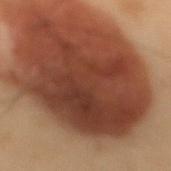| key | value |
|---|---|
| anatomic site | the mid back |
| automated metrics | a shape-asymmetry score of about 0.3 (0 = symmetric); a border-irregularity rating of about 4.5/10, a color-variation rating of about 5/10, and peripheral color asymmetry of about 1.5; a nevus-likeness score of about 70/100 and a lesion-detection confidence of about 55/100 |
| subject | male, in their mid-50s |
| acquisition | 15 mm crop, total-body photography |
| lesion size | ~13 mm (longest diameter) |